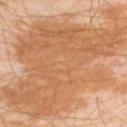Impression: Imaged during a routine full-body skin examination; the lesion was not biopsied and no histopathology is available. Acquisition and patient details: Automated image analysis of the tile measured a detector confidence of about 95 out of 100 that the crop contains a lesion. Measured at roughly 18.5 mm in maximum diameter. The tile uses cross-polarized illumination. The subject is a male approximately 30 years of age. Located on the leg. A 15 mm crop from a total-body photograph taken for skin-cancer surveillance.